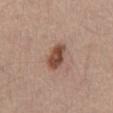Impression: No biopsy was performed on this lesion — it was imaged during a full skin examination and was not determined to be concerning. Clinical summary: A male patient aged 68 to 72. A 15 mm close-up tile from a total-body photography series done for melanoma screening. The lesion is on the abdomen.